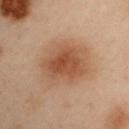follow-up: catalogued during a skin exam; not biopsied
image source: ~15 mm tile from a whole-body skin photo
diameter: about 6 mm
automated metrics: a footprint of about 21 mm² and a shape-asymmetry score of about 0.2 (0 = symmetric); a mean CIELAB color near L≈41 a*≈18 b*≈27 and roughly 9 lightness units darker than nearby skin
site: the right upper arm
tile lighting: cross-polarized illumination
subject: male, in their mid- to late 50s